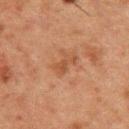| field | value |
|---|---|
| biopsy status | imaged on a skin check; not biopsied |
| tile lighting | cross-polarized |
| body site | the upper back |
| patient | male, aged 48 to 52 |
| automated lesion analysis | a lesion color around L≈39 a*≈20 b*≈29 in CIELAB, a lesion–skin lightness drop of about 6, and a normalized lesion–skin contrast near 6; a border-irregularity rating of about 7/10, internal color variation of about 0 on a 0–10 scale, and radial color variation of about 0 |
| image | ~15 mm crop, total-body skin-cancer survey |
| size | ≈3 mm |